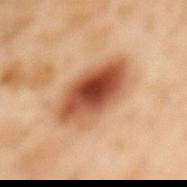Q: Was this lesion biopsied?
A: no biopsy performed (imaged during a skin exam)
Q: How was this image acquired?
A: 15 mm crop, total-body photography
Q: Lesion location?
A: the back
Q: Patient demographics?
A: female, approximately 55 years of age